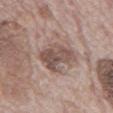A male patient roughly 70 years of age. Imaged with white-light lighting. Measured at roughly 4.5 mm in maximum diameter. On the abdomen. A lesion tile, about 15 mm wide, cut from a 3D total-body photograph.